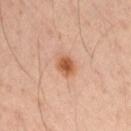Impression: This lesion was catalogued during total-body skin photography and was not selected for biopsy. Image and clinical context: A lesion tile, about 15 mm wide, cut from a 3D total-body photograph. About 2.5 mm across. The lesion is on the arm. A male subject approximately 35 years of age. Imaged with cross-polarized lighting.